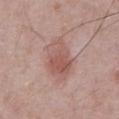The lesion was tiled from a total-body skin photograph and was not biopsied.
The patient is a male aged around 65.
The lesion is located on the abdomen.
The tile uses white-light illumination.
A lesion tile, about 15 mm wide, cut from a 3D total-body photograph.
Longest diameter approximately 5 mm.